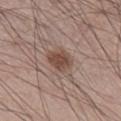The lesion was tiled from a total-body skin photograph and was not biopsied.
The tile uses white-light illumination.
Automated image analysis of the tile measured a shape eccentricity near 0.6. The software also gave a border-irregularity index near 2/10, internal color variation of about 2.5 on a 0–10 scale, and radial color variation of about 1.
The lesion's longest dimension is about 3 mm.
A 15 mm close-up extracted from a 3D total-body photography capture.
The subject is a male aged around 60.
The lesion is on the right lower leg.